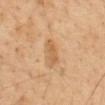  biopsy_status: not biopsied; imaged during a skin examination
  lesion_size:
    long_diameter_mm_approx: 3.5
  image:
    source: total-body photography crop
    field_of_view_mm: 15
  patient:
    sex: male
    age_approx: 50
  automated_metrics:
    cielab_L: 59
    cielab_a: 20
    cielab_b: 40
    vs_skin_darker_L: 9.0
    vs_skin_contrast_norm: 6.5
    nevus_likeness_0_100: 5
    lesion_detection_confidence_0_100: 100
  site: abdomen